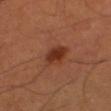Q: Is there a histopathology result?
A: no biopsy performed (imaged during a skin exam)
Q: What is the anatomic site?
A: the right thigh
Q: How was this image acquired?
A: ~15 mm crop, total-body skin-cancer survey
Q: What are the patient's age and sex?
A: male, roughly 50 years of age
Q: Illumination type?
A: cross-polarized